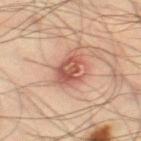Assessment: Captured during whole-body skin photography for melanoma surveillance; the lesion was not biopsied. Context: About 5 mm across. A lesion tile, about 15 mm wide, cut from a 3D total-body photograph. From the right thigh. The patient is a male approximately 35 years of age. Captured under cross-polarized illumination. The total-body-photography lesion software estimated a shape eccentricity near 0.75 and two-axis asymmetry of about 0.25. And it measured a mean CIELAB color near L≈51 a*≈23 b*≈27, a lesion–skin lightness drop of about 12, and a lesion-to-skin contrast of about 8 (normalized; higher = more distinct). The analysis additionally found a classifier nevus-likeness of about 5/100.